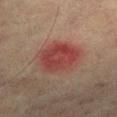Imaged during a routine full-body skin examination; the lesion was not biopsied and no histopathology is available. Automated tile analysis of the lesion measured an eccentricity of roughly 0.5 and two-axis asymmetry of about 0.15. It also reported a lesion color around L≈36 a*≈24 b*≈22 in CIELAB, a lesion–skin lightness drop of about 8, and a normalized lesion–skin contrast near 7.5. It also reported a color-variation rating of about 5/10 and peripheral color asymmetry of about 2. The recorded lesion diameter is about 5 mm. The tile uses cross-polarized illumination. Located on the left lower leg. A male subject approximately 75 years of age. A close-up tile cropped from a whole-body skin photograph, about 15 mm across.